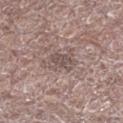Impression:
Part of a total-body skin-imaging series; this lesion was reviewed on a skin check and was not flagged for biopsy.
Acquisition and patient details:
Approximately 3.5 mm at its widest. The tile uses white-light illumination. The subject is a male approximately 70 years of age. The lesion is located on the left lower leg. Cropped from a total-body skin-imaging series; the visible field is about 15 mm.